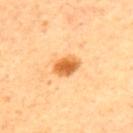{"biopsy_status": "not biopsied; imaged during a skin examination", "lighting": "cross-polarized", "patient": {"sex": "male", "age_approx": 60}, "automated_metrics": {"area_mm2_approx": 6.0, "eccentricity": 0.7, "cielab_L": 64, "cielab_a": 28, "cielab_b": 49, "vs_skin_contrast_norm": 9.5, "border_irregularity_0_10": 2.0, "color_variation_0_10": 3.5, "nevus_likeness_0_100": 100, "lesion_detection_confidence_0_100": 100}, "site": "upper back", "image": {"source": "total-body photography crop", "field_of_view_mm": 15}}